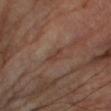– workup: imaged on a skin check; not biopsied
– body site: the right upper arm
– tile lighting: cross-polarized illumination
– imaging modality: total-body-photography crop, ~15 mm field of view
– subject: male, aged around 70
– diameter: about 2.5 mm
– TBP lesion metrics: a lesion area of about 3 mm², an outline eccentricity of about 0.85 (0 = round, 1 = elongated), and a symmetry-axis asymmetry near 0.25; a classifier nevus-likeness of about 0/100 and lesion-presence confidence of about 85/100